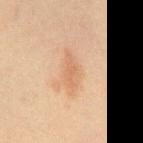Impression:
Captured during whole-body skin photography for melanoma surveillance; the lesion was not biopsied.
Acquisition and patient details:
Approximately 4 mm at its widest. Located on the abdomen. A male subject, approximately 60 years of age. Captured under cross-polarized illumination. A region of skin cropped from a whole-body photographic capture, roughly 15 mm wide. An algorithmic analysis of the crop reported a footprint of about 4.5 mm², an eccentricity of roughly 0.95, and a symmetry-axis asymmetry near 0.3. The analysis additionally found an average lesion color of about L≈53 a*≈18 b*≈31 (CIELAB) and a normalized border contrast of about 5.5. And it measured an automated nevus-likeness rating near 0 out of 100 and a lesion-detection confidence of about 100/100.